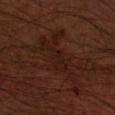| key | value |
|---|---|
| follow-up | imaged on a skin check; not biopsied |
| site | the right forearm |
| image-analysis metrics | a lesion-to-skin contrast of about 5.5 (normalized; higher = more distinct) |
| image | ~15 mm tile from a whole-body skin photo |
| patient | male, aged approximately 60 |
| size | about 9 mm |
| tile lighting | cross-polarized illumination |